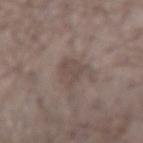notes = catalogued during a skin exam; not biopsied
subject = male, about 55 years old
size = ≈4 mm
site = the right forearm
image source = ~15 mm crop, total-body skin-cancer survey